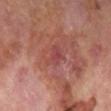lighting: cross-polarized illumination; image source: ~15 mm tile from a whole-body skin photo; patient: male, aged approximately 70; lesion diameter: ≈3 mm; site: the left lower leg.